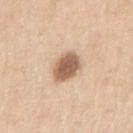Assessment: Part of a total-body skin-imaging series; this lesion was reviewed on a skin check and was not flagged for biopsy. Image and clinical context: Automated image analysis of the tile measured a mean CIELAB color near L≈60 a*≈18 b*≈31, roughly 16 lightness units darker than nearby skin, and a normalized lesion–skin contrast near 10. The analysis additionally found a border-irregularity rating of about 2/10 and a peripheral color-asymmetry measure near 1. The lesion is located on the right upper arm. A male patient aged around 60. A close-up tile cropped from a whole-body skin photograph, about 15 mm across. Approximately 3.5 mm at its widest.